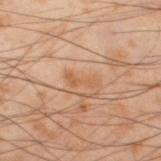{
  "biopsy_status": "not biopsied; imaged during a skin examination",
  "site": "left thigh",
  "image": {
    "source": "total-body photography crop",
    "field_of_view_mm": 15
  },
  "patient": {
    "sex": "male",
    "age_approx": 55
  }
}